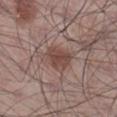Captured during whole-body skin photography for melanoma surveillance; the lesion was not biopsied. A 15 mm crop from a total-body photograph taken for skin-cancer surveillance. The lesion is located on the right lower leg. Longest diameter approximately 3.5 mm. A male subject, roughly 55 years of age. The tile uses white-light illumination.